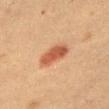patient = female, aged 38–42
lesion size = ~4.5 mm (longest diameter)
lighting = cross-polarized illumination
imaging modality = 15 mm crop, total-body photography
body site = the right thigh